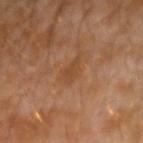biopsy status: no biopsy performed (imaged during a skin exam); location: the left arm; tile lighting: cross-polarized; subject: male, about 30 years old; automated lesion analysis: a footprint of about 3.5 mm², a shape eccentricity near 0.85, and two-axis asymmetry of about 0.3; image source: 15 mm crop, total-body photography.